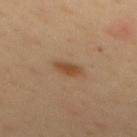Q: Is there a histopathology result?
A: imaged on a skin check; not biopsied
Q: Lesion size?
A: about 3 mm
Q: Where on the body is the lesion?
A: the mid back
Q: What kind of image is this?
A: ~15 mm tile from a whole-body skin photo
Q: Illumination type?
A: cross-polarized
Q: What are the patient's age and sex?
A: female, approximately 50 years of age